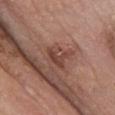follow-up: total-body-photography surveillance lesion; no biopsy
lesion diameter: about 3 mm
patient: male, aged around 75
TBP lesion metrics: a mean CIELAB color near L≈42 a*≈22 b*≈25; border irregularity of about 7 on a 0–10 scale
illumination: white-light
anatomic site: the abdomen
image: ~15 mm crop, total-body skin-cancer survey